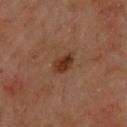The lesion was tiled from a total-body skin photograph and was not biopsied.